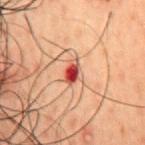Assessment: No biopsy was performed on this lesion — it was imaged during a full skin examination and was not determined to be concerning. Context: Measured at roughly 3 mm in maximum diameter. This is a cross-polarized tile. From the chest. A male subject, in their 50s. A roughly 15 mm field-of-view crop from a total-body skin photograph. An algorithmic analysis of the crop reported a border-irregularity index near 2/10 and a color-variation rating of about 7.5/10. It also reported an automated nevus-likeness rating near 0 out of 100 and a lesion-detection confidence of about 100/100.